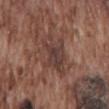The lesion was photographed on a routine skin check and not biopsied; there is no pathology result.